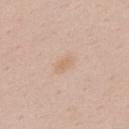Part of a total-body skin-imaging series; this lesion was reviewed on a skin check and was not flagged for biopsy. A male patient aged approximately 50. This is a white-light tile. A roughly 15 mm field-of-view crop from a total-body skin photograph. The lesion is located on the upper back. Measured at roughly 3 mm in maximum diameter.